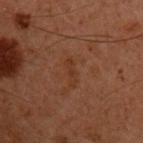Imaged during a routine full-body skin examination; the lesion was not biopsied and no histopathology is available. The subject is a male in their 60s. About 3 mm across. An algorithmic analysis of the crop reported a footprint of about 2 mm², a shape eccentricity near 0.95, and a shape-asymmetry score of about 0.4 (0 = symmetric). The analysis additionally found a mean CIELAB color near L≈25 a*≈18 b*≈24 and about 4 CIELAB-L* units darker than the surrounding skin. This is a cross-polarized tile. Cropped from a whole-body photographic skin survey; the tile spans about 15 mm. From the front of the torso.